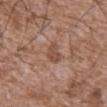Notes:
– subject: male, roughly 75 years of age
– tile lighting: white-light
– automated lesion analysis: an area of roughly 4 mm², a shape eccentricity near 0.85, and a symmetry-axis asymmetry near 0.25; a mean CIELAB color near L≈49 a*≈20 b*≈28 and a normalized lesion–skin contrast near 6.5
– body site: the abdomen
– image: 15 mm crop, total-body photography
– diameter: about 3 mm